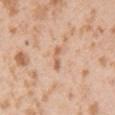The lesion was tiled from a total-body skin photograph and was not biopsied. The recorded lesion diameter is about 2.5 mm. A roughly 15 mm field-of-view crop from a total-body skin photograph. The patient is a male in their mid- to late 20s. On the left upper arm.